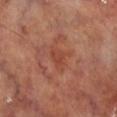Clinical impression:
No biopsy was performed on this lesion — it was imaged during a full skin examination and was not determined to be concerning.